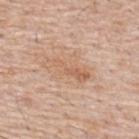{"site": "upper back", "image": {"source": "total-body photography crop", "field_of_view_mm": 15}, "lighting": "white-light", "lesion_size": {"long_diameter_mm_approx": 6.0}, "patient": {"sex": "male", "age_approx": 55}}